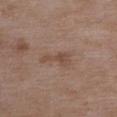Impression: Part of a total-body skin-imaging series; this lesion was reviewed on a skin check and was not flagged for biopsy. Acquisition and patient details: Captured under white-light illumination. The lesion's longest dimension is about 3.5 mm. A roughly 15 mm field-of-view crop from a total-body skin photograph. From the upper back. A female patient aged 68–72.